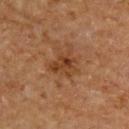Case summary:
• follow-up · imaged on a skin check; not biopsied
• illumination · cross-polarized illumination
• location · the upper back
• diameter · about 3.5 mm
• acquisition · total-body-photography crop, ~15 mm field of view
• subject · male, in their mid- to late 60s